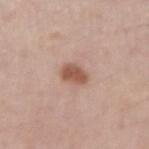The lesion was tiled from a total-body skin photograph and was not biopsied. Longest diameter approximately 2.5 mm. Automated tile analysis of the lesion measured a nevus-likeness score of about 95/100 and lesion-presence confidence of about 100/100. Cropped from a whole-body photographic skin survey; the tile spans about 15 mm. A male subject, in their 60s. From the right upper arm. The tile uses white-light illumination.